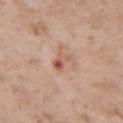Longest diameter approximately 2.5 mm.
From the right upper arm.
Cropped from a total-body skin-imaging series; the visible field is about 15 mm.
A male patient, approximately 50 years of age.
Automated tile analysis of the lesion measured an area of roughly 3.5 mm², a shape eccentricity near 0.8, and a shape-asymmetry score of about 0.35 (0 = symmetric). And it measured a lesion color around L≈57 a*≈24 b*≈28 in CIELAB, about 10 CIELAB-L* units darker than the surrounding skin, and a lesion-to-skin contrast of about 7 (normalized; higher = more distinct). And it measured a border-irregularity index near 4/10, internal color variation of about 8 on a 0–10 scale, and a peripheral color-asymmetry measure near 2. And it measured a classifier nevus-likeness of about 0/100 and lesion-presence confidence of about 100/100.